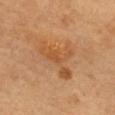Impression: This lesion was catalogued during total-body skin photography and was not selected for biopsy. Background: On the upper back. A female subject, in their 60s. This is a cross-polarized tile. A 15 mm close-up extracted from a 3D total-body photography capture. Longest diameter approximately 7 mm.